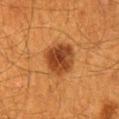Recorded during total-body skin imaging; not selected for excision or biopsy.
A male subject, aged approximately 60.
A close-up tile cropped from a whole-body skin photograph, about 15 mm across.
On the back.